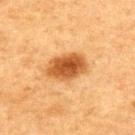The lesion was photographed on a routine skin check and not biopsied; there is no pathology result. A 15 mm crop from a total-body photograph taken for skin-cancer surveillance. Automated image analysis of the tile measured a footprint of about 12 mm², an outline eccentricity of about 0.8 (0 = round, 1 = elongated), and two-axis asymmetry of about 0.15. The software also gave an average lesion color of about L≈45 a*≈23 b*≈39 (CIELAB) and roughly 14 lightness units darker than nearby skin. The analysis additionally found a border-irregularity index near 1.5/10, internal color variation of about 4.5 on a 0–10 scale, and a peripheral color-asymmetry measure near 1.5. The software also gave a classifier nevus-likeness of about 100/100 and a detector confidence of about 100 out of 100 that the crop contains a lesion. On the upper back. A male patient aged 73–77. Captured under cross-polarized illumination. Approximately 5 mm at its widest.